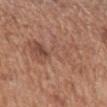Clinical impression: Imaged during a routine full-body skin examination; the lesion was not biopsied and no histopathology is available. Context: A male subject, in their mid-60s. Captured under white-light illumination. Measured at roughly 8 mm in maximum diameter. Cropped from a total-body skin-imaging series; the visible field is about 15 mm. The lesion is on the arm.